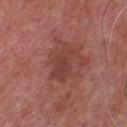Assessment: The lesion was photographed on a routine skin check and not biopsied; there is no pathology result. Acquisition and patient details: A 15 mm crop from a total-body photograph taken for skin-cancer surveillance. The patient is a male aged 63–67. This is a white-light tile. On the chest. Approximately 4.5 mm at its widest.